On the right lower leg · a female patient, roughly 65 years of age · a 15 mm close-up extracted from a 3D total-body photography capture:
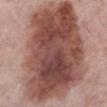Measured at roughly 13 mm in maximum diameter.
This is a white-light tile.
The lesion was biopsied, and histopathology showed a malignancy: melanoma in situ.